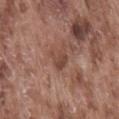No biopsy was performed on this lesion — it was imaged during a full skin examination and was not determined to be concerning.
Cropped from a whole-body photographic skin survey; the tile spans about 15 mm.
Automated image analysis of the tile measured a lesion area of about 4.5 mm². It also reported a mean CIELAB color near L≈45 a*≈21 b*≈26 and a lesion–skin lightness drop of about 8. The software also gave a color-variation rating of about 3/10 and a peripheral color-asymmetry measure near 1. It also reported an automated nevus-likeness rating near 0 out of 100 and a detector confidence of about 100 out of 100 that the crop contains a lesion.
Captured under white-light illumination.
The recorded lesion diameter is about 2.5 mm.
A male subject, roughly 75 years of age.
The lesion is located on the lower back.